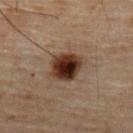Clinical impression: Imaged during a routine full-body skin examination; the lesion was not biopsied and no histopathology is available. Context: The subject is a male aged around 70. Longest diameter approximately 3.5 mm. Imaged with cross-polarized lighting. The lesion is on the abdomen. A close-up tile cropped from a whole-body skin photograph, about 15 mm across.